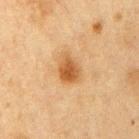biopsy status — catalogued during a skin exam; not biopsied
site — the arm
illumination — cross-polarized illumination
subject — female, roughly 40 years of age
image source — total-body-photography crop, ~15 mm field of view
size — about 3.5 mm
TBP lesion metrics — a shape-asymmetry score of about 0.25 (0 = symmetric); an average lesion color of about L≈50 a*≈20 b*≈38 (CIELAB), roughly 11 lightness units darker than nearby skin, and a lesion-to-skin contrast of about 8.5 (normalized; higher = more distinct); a nevus-likeness score of about 95/100